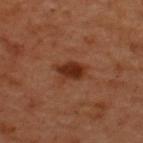notes = total-body-photography surveillance lesion; no biopsy
location = the back
lighting = cross-polarized
patient = male, in their 50s
imaging modality = ~15 mm crop, total-body skin-cancer survey